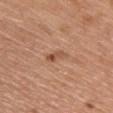<record>
  <biopsy_status>not biopsied; imaged during a skin examination</biopsy_status>
  <lighting>white-light</lighting>
  <image>
    <source>total-body photography crop</source>
    <field_of_view_mm>15</field_of_view_mm>
  </image>
  <patient>
    <sex>female</sex>
    <age_approx>60</age_approx>
  </patient>
  <lesion_size>
    <long_diameter_mm_approx>3.0</long_diameter_mm_approx>
  </lesion_size>
  <site>chest</site>
</record>